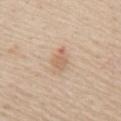| feature | finding |
|---|---|
| biopsy status | total-body-photography surveillance lesion; no biopsy |
| illumination | white-light |
| patient | male, aged 58–62 |
| location | the mid back |
| TBP lesion metrics | a mean CIELAB color near L≈63 a*≈18 b*≈31, a lesion–skin lightness drop of about 9, and a lesion-to-skin contrast of about 6 (normalized; higher = more distinct); border irregularity of about 3.5 on a 0–10 scale, a within-lesion color-variation index near 1.5/10, and a peripheral color-asymmetry measure near 0.5; a classifier nevus-likeness of about 0/100 |
| imaging modality | total-body-photography crop, ~15 mm field of view |
| lesion size | about 3 mm |